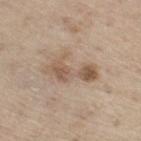Background: Imaged with white-light lighting. The recorded lesion diameter is about 6 mm. A male subject, in their 70s. A close-up tile cropped from a whole-body skin photograph, about 15 mm across. The lesion is located on the leg.